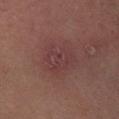Findings:
* workup · total-body-photography surveillance lesion; no biopsy